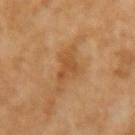Context: A female subject, aged approximately 70. Automated image analysis of the tile measured a classifier nevus-likeness of about 0/100 and a detector confidence of about 100 out of 100 that the crop contains a lesion. Located on the right forearm. Cropped from a total-body skin-imaging series; the visible field is about 15 mm. Approximately 4.5 mm at its widest.